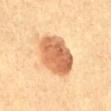Impression: The lesion was tiled from a total-body skin photograph and was not biopsied. Clinical summary: A 15 mm close-up extracted from a 3D total-body photography capture. This is a cross-polarized tile. From the abdomen. A female subject, aged approximately 55. Longest diameter approximately 5.5 mm.